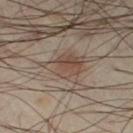follow-up = catalogued during a skin exam; not biopsied | image = ~15 mm crop, total-body skin-cancer survey | automated metrics = a footprint of about 12 mm² and a shape eccentricity near 0.65; an average lesion color of about L≈47 a*≈14 b*≈24 (CIELAB) and a normalized border contrast of about 6 | subject = male, aged 38–42 | body site = the left thigh | size = ≈5 mm.